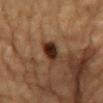Imaged during a routine full-body skin examination; the lesion was not biopsied and no histopathology is available. A male subject in their mid-80s or older. Approximately 4 mm at its widest. A 15 mm crop from a total-body photograph taken for skin-cancer surveillance. The lesion-visualizer software estimated border irregularity of about 2.5 on a 0–10 scale, internal color variation of about 4.5 on a 0–10 scale, and peripheral color asymmetry of about 1.5. The software also gave an automated nevus-likeness rating near 95 out of 100 and a lesion-detection confidence of about 100/100. On the chest.